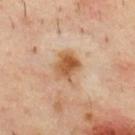Impression:
The lesion was tiled from a total-body skin photograph and was not biopsied.
Image and clinical context:
Imaged with cross-polarized lighting. From the upper back. A 15 mm crop from a total-body photograph taken for skin-cancer surveillance. A male subject in their 40s. An algorithmic analysis of the crop reported a detector confidence of about 100 out of 100 that the crop contains a lesion. About 4 mm across.